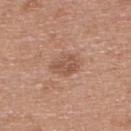This lesion was catalogued during total-body skin photography and was not selected for biopsy.
The lesion is on the upper back.
Captured under white-light illumination.
A close-up tile cropped from a whole-body skin photograph, about 15 mm across.
A male subject, aged approximately 30.
Automated tile analysis of the lesion measured a footprint of about 5 mm² and a shape eccentricity near 0.65. It also reported an average lesion color of about L≈53 a*≈22 b*≈29 (CIELAB). The analysis additionally found a classifier nevus-likeness of about 15/100 and a detector confidence of about 100 out of 100 that the crop contains a lesion.